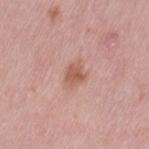Imaged during a routine full-body skin examination; the lesion was not biopsied and no histopathology is available.
The recorded lesion diameter is about 3 mm.
On the left thigh.
The subject is a female roughly 40 years of age.
A close-up tile cropped from a whole-body skin photograph, about 15 mm across.
An algorithmic analysis of the crop reported an area of roughly 5 mm², a shape eccentricity near 0.6, and two-axis asymmetry of about 0.3. The analysis additionally found a lesion color around L≈57 a*≈24 b*≈28 in CIELAB, roughly 10 lightness units darker than nearby skin, and a normalized lesion–skin contrast near 7. And it measured border irregularity of about 3 on a 0–10 scale and a color-variation rating of about 2.5/10.